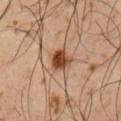No biopsy was performed on this lesion — it was imaged during a full skin examination and was not determined to be concerning.
Cropped from a whole-body photographic skin survey; the tile spans about 15 mm.
On the arm.
Imaged with cross-polarized lighting.
A male patient aged 48 to 52.
The recorded lesion diameter is about 3 mm.